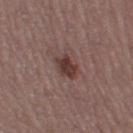workup = imaged on a skin check; not biopsied | automated lesion analysis = a lesion area of about 6 mm² and an eccentricity of roughly 0.8; a border-irregularity rating of about 2/10, internal color variation of about 3.5 on a 0–10 scale, and a peripheral color-asymmetry measure near 1 | diameter = ~3.5 mm (longest diameter) | lighting = white-light illumination | site = the leg | image source = 15 mm crop, total-body photography | patient = female, aged around 55.